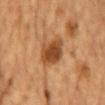This lesion was catalogued during total-body skin photography and was not selected for biopsy. A male patient, aged 83 or over. Measured at roughly 4 mm in maximum diameter. On the chest. The tile uses cross-polarized illumination. A roughly 15 mm field-of-view crop from a total-body skin photograph. Automated image analysis of the tile measured an area of roughly 9 mm², an outline eccentricity of about 0.75 (0 = round, 1 = elongated), and a symmetry-axis asymmetry near 0.2. The analysis additionally found a lesion color around L≈40 a*≈21 b*≈34 in CIELAB and roughly 12 lightness units darker than nearby skin. It also reported a border-irregularity index near 2/10, internal color variation of about 4 on a 0–10 scale, and peripheral color asymmetry of about 1. The software also gave a nevus-likeness score of about 95/100 and a detector confidence of about 100 out of 100 that the crop contains a lesion.